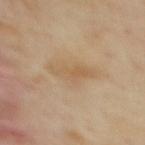follow-up: imaged on a skin check; not biopsied | subject: female, aged approximately 40 | site: the upper back | image source: ~15 mm tile from a whole-body skin photo.